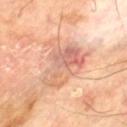follow-up=catalogued during a skin exam; not biopsied
image=~15 mm crop, total-body skin-cancer survey
patient=male, roughly 70 years of age
site=the left thigh